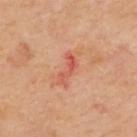Notes:
– workup · catalogued during a skin exam; not biopsied
– subject · female, about 40 years old
– lesion diameter · about 3.5 mm
– imaging modality · 15 mm crop, total-body photography
– site · the back